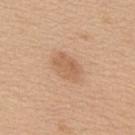Impression: Recorded during total-body skin imaging; not selected for excision or biopsy. Context: A 15 mm close-up extracted from a 3D total-body photography capture. Measured at roughly 4 mm in maximum diameter. Imaged with white-light lighting. A male patient aged approximately 60. On the upper back. An algorithmic analysis of the crop reported an average lesion color of about L≈61 a*≈20 b*≈34 (CIELAB) and a normalized lesion–skin contrast near 5.5. It also reported a classifier nevus-likeness of about 35/100.